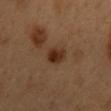- biopsy status: catalogued during a skin exam; not biopsied
- patient: male, aged approximately 50
- site: the mid back
- lesion size: about 2.5 mm
- automated lesion analysis: an average lesion color of about L≈30 a*≈19 b*≈29 (CIELAB), a lesion–skin lightness drop of about 10, and a lesion-to-skin contrast of about 10 (normalized; higher = more distinct); a border-irregularity index near 2.5/10, a color-variation rating of about 4.5/10, and a peripheral color-asymmetry measure near 1.5
- illumination: cross-polarized illumination
- acquisition: ~15 mm crop, total-body skin-cancer survey